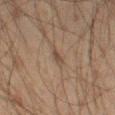biopsy_status: not biopsied; imaged during a skin examination
image:
  source: total-body photography crop
  field_of_view_mm: 15
site: left thigh
patient:
  sex: male
  age_approx: 60